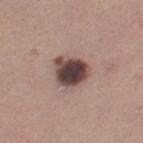Imaged during a routine full-body skin examination; the lesion was not biopsied and no histopathology is available. Measured at roughly 4 mm in maximum diameter. The subject is a female aged 28–32. An algorithmic analysis of the crop reported an area of roughly 11 mm² and an eccentricity of roughly 0.4. The analysis additionally found a lesion color around L≈43 a*≈17 b*≈19 in CIELAB. The lesion is on the left thigh. A region of skin cropped from a whole-body photographic capture, roughly 15 mm wide.